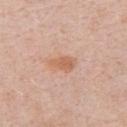workup — no biopsy performed (imaged during a skin exam)
location — the chest
size — about 3 mm
imaging modality — ~15 mm tile from a whole-body skin photo
patient — male, aged 68 to 72
automated lesion analysis — border irregularity of about 2.5 on a 0–10 scale, a color-variation rating of about 2/10, and peripheral color asymmetry of about 0.5
illumination — white-light